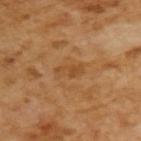Recorded during total-body skin imaging; not selected for excision or biopsy. A close-up tile cropped from a whole-body skin photograph, about 15 mm across. The subject is a male aged 63 to 67. An algorithmic analysis of the crop reported an area of roughly 4.5 mm², an eccentricity of roughly 0.9, and a shape-asymmetry score of about 0.2 (0 = symmetric). The software also gave an average lesion color of about L≈46 a*≈20 b*≈38 (CIELAB), about 6 CIELAB-L* units darker than the surrounding skin, and a lesion-to-skin contrast of about 5 (normalized; higher = more distinct). The recorded lesion diameter is about 3.5 mm. Captured under cross-polarized illumination.